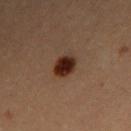| feature | finding |
|---|---|
| notes | imaged on a skin check; not biopsied |
| size | ~3 mm (longest diameter) |
| acquisition | 15 mm crop, total-body photography |
| subject | female, in their 30s |
| site | the left arm |
| lighting | cross-polarized illumination |